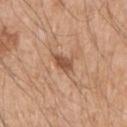<case>
  <biopsy_status>not biopsied; imaged during a skin examination</biopsy_status>
  <image>
    <source>total-body photography crop</source>
    <field_of_view_mm>15</field_of_view_mm>
  </image>
  <automated_metrics>
    <area_mm2_approx>4.0</area_mm2_approx>
    <eccentricity>0.7</eccentricity>
    <shape_asymmetry>0.25</shape_asymmetry>
    <nevus_likeness_0_100>70</nevus_likeness_0_100>
    <lesion_detection_confidence_0_100>100</lesion_detection_confidence_0_100>
  </automated_metrics>
  <patient>
    <sex>male</sex>
    <age_approx>50</age_approx>
  </patient>
  <lighting>white-light</lighting>
  <site>left upper arm</site>
  <lesion_size>
    <long_diameter_mm_approx>2.5</long_diameter_mm_approx>
  </lesion_size>
</case>